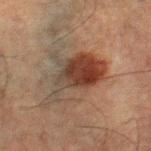Part of a total-body skin-imaging series; this lesion was reviewed on a skin check and was not flagged for biopsy.
Captured under cross-polarized illumination.
The patient is a male aged 48 to 52.
Measured at roughly 8 mm in maximum diameter.
The lesion is on the leg.
Cropped from a total-body skin-imaging series; the visible field is about 15 mm.